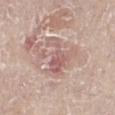biopsy_status: not biopsied; imaged during a skin examination
lighting: white-light
lesion_size:
  long_diameter_mm_approx: 5.0
patient:
  sex: female
  age_approx: 70
site: right lower leg
image:
  source: total-body photography crop
  field_of_view_mm: 15